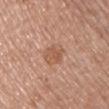The lesion was tiled from a total-body skin photograph and was not biopsied. Automated image analysis of the tile measured a footprint of about 7 mm², an outline eccentricity of about 0.35 (0 = round, 1 = elongated), and a shape-asymmetry score of about 0.25 (0 = symmetric). The analysis additionally found an average lesion color of about L≈56 a*≈21 b*≈32 (CIELAB), a lesion–skin lightness drop of about 7, and a lesion-to-skin contrast of about 5.5 (normalized; higher = more distinct). The subject is a female in their 50s. The recorded lesion diameter is about 3 mm. The lesion is on the chest. A roughly 15 mm field-of-view crop from a total-body skin photograph. Captured under white-light illumination.